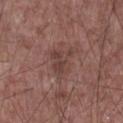No biopsy was performed on this lesion — it was imaged during a full skin examination and was not determined to be concerning.